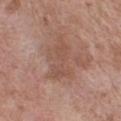Impression: Recorded during total-body skin imaging; not selected for excision or biopsy. Context: Cropped from a whole-body photographic skin survey; the tile spans about 15 mm. This is a white-light tile. Longest diameter approximately 5 mm. Located on the chest. A male subject aged 48 to 52.